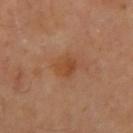| field | value |
|---|---|
| notes | imaged on a skin check; not biopsied |
| illumination | cross-polarized illumination |
| image | ~15 mm tile from a whole-body skin photo |
| site | the arm |